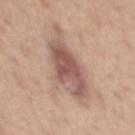| key | value |
|---|---|
| image-analysis metrics | a lesion color around L≈56 a*≈19 b*≈24 in CIELAB and a normalized lesion–skin contrast near 8.5; a border-irregularity index near 4.5/10, a within-lesion color-variation index near 6/10, and a peripheral color-asymmetry measure near 2 |
| location | the back |
| lighting | white-light |
| diameter | ≈9 mm |
| image source | ~15 mm tile from a whole-body skin photo |
| subject | male, aged 38–42 |